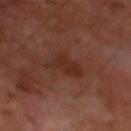<lesion>
<patient>
  <sex>male</sex>
  <age_approx>70</age_approx>
</patient>
<site>left lower leg</site>
<lighting>cross-polarized</lighting>
<automated_metrics>
  <eccentricity>0.7</eccentricity>
  <shape_asymmetry>0.45</shape_asymmetry>
  <nevus_likeness_0_100>0</nevus_likeness_0_100>
  <lesion_detection_confidence_0_100>100</lesion_detection_confidence_0_100>
</automated_metrics>
<image>
  <source>total-body photography crop</source>
  <field_of_view_mm>15</field_of_view_mm>
</image>
</lesion>